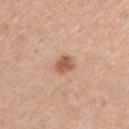biopsy status = total-body-photography surveillance lesion; no biopsy
subject = male, aged around 50
image-analysis metrics = a mean CIELAB color near L≈57 a*≈22 b*≈32 and a lesion–skin lightness drop of about 12; an automated nevus-likeness rating near 90 out of 100
image = ~15 mm tile from a whole-body skin photo
lesion diameter = ~2.5 mm (longest diameter)
location = the right upper arm
illumination = white-light illumination